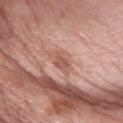No biopsy was performed on this lesion — it was imaged during a full skin examination and was not determined to be concerning.
A female patient aged approximately 60.
This image is a 15 mm lesion crop taken from a total-body photograph.
This is a white-light tile.
Longest diameter approximately 2.5 mm.
The lesion is on the right forearm.